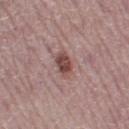Impression: Recorded during total-body skin imaging; not selected for excision or biopsy. Clinical summary: A lesion tile, about 15 mm wide, cut from a 3D total-body photograph. About 3 mm across. Located on the right thigh. A female patient roughly 50 years of age.